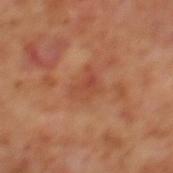{"biopsy_status": "not biopsied; imaged during a skin examination", "image": {"source": "total-body photography crop", "field_of_view_mm": 15}, "site": "mid back", "patient": {"sex": "male", "age_approx": 70}, "lesion_size": {"long_diameter_mm_approx": 3.0}, "lighting": "cross-polarized", "automated_metrics": {"cielab_L": 45, "cielab_a": 26, "cielab_b": 32, "vs_skin_darker_L": 6.0, "vs_skin_contrast_norm": 5.0, "peripheral_color_asymmetry": 1.0, "lesion_detection_confidence_0_100": 100}}